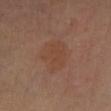A female subject aged approximately 60.
Located on the left lower leg.
About 5 mm across.
Captured under cross-polarized illumination.
A 15 mm close-up tile from a total-body photography series done for melanoma screening.